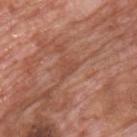biopsy status: no biopsy performed (imaged during a skin exam) | acquisition: total-body-photography crop, ~15 mm field of view | body site: the upper back | subject: male, aged approximately 75 | lighting: white-light.